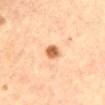The lesion was tiled from a total-body skin photograph and was not biopsied.
The lesion-visualizer software estimated a lesion color around L≈63 a*≈23 b*≈39 in CIELAB and a normalized border contrast of about 9.5.
Located on the chest.
Imaged with cross-polarized lighting.
A female patient, about 65 years old.
Cropped from a total-body skin-imaging series; the visible field is about 15 mm.